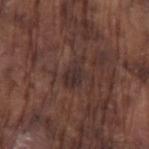<lesion>
<biopsy_status>not biopsied; imaged during a skin examination</biopsy_status>
<patient>
  <sex>male</sex>
  <age_approx>75</age_approx>
</patient>
<image>
  <source>total-body photography crop</source>
  <field_of_view_mm>15</field_of_view_mm>
</image>
<lighting>white-light</lighting>
<lesion_size>
  <long_diameter_mm_approx>3.5</long_diameter_mm_approx>
</lesion_size>
<site>left upper arm</site>
</lesion>